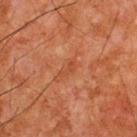This lesion was catalogued during total-body skin photography and was not selected for biopsy.
Longest diameter approximately 2.5 mm.
Cropped from a whole-body photographic skin survey; the tile spans about 15 mm.
Automated image analysis of the tile measured an outline eccentricity of about 0.9 (0 = round, 1 = elongated) and two-axis asymmetry of about 0.4.
Captured under cross-polarized illumination.
The patient is a male about 65 years old.
The lesion is located on the back.